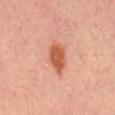Background:
This image is a 15 mm lesion crop taken from a total-body photograph. Located on the mid back. Imaged with cross-polarized lighting. Longest diameter approximately 4 mm. A male subject aged 28–32.